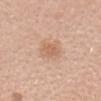follow-up: catalogued during a skin exam; not biopsied
site: the head or neck
size: ≈3 mm
image source: ~15 mm tile from a whole-body skin photo
patient: female, aged 38 to 42
TBP lesion metrics: a footprint of about 4 mm², a shape eccentricity near 0.75, and a shape-asymmetry score of about 0.3 (0 = symmetric); a lesion color around L≈62 a*≈20 b*≈32 in CIELAB, a lesion–skin lightness drop of about 8, and a lesion-to-skin contrast of about 5.5 (normalized; higher = more distinct); a border-irregularity index near 3/10 and peripheral color asymmetry of about 0.5